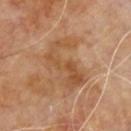Findings:
* follow-up — catalogued during a skin exam; not biopsied
* imaging modality — 15 mm crop, total-body photography
* site — the upper back
* image-analysis metrics — a footprint of about 16 mm², an eccentricity of roughly 0.85, and a shape-asymmetry score of about 0.5 (0 = symmetric); a lesion color around L≈49 a*≈20 b*≈34 in CIELAB and about 7 CIELAB-L* units darker than the surrounding skin; lesion-presence confidence of about 100/100
* subject — male, aged 58–62